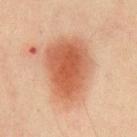Notes:
* follow-up: no biopsy performed (imaged during a skin exam)
* lesion diameter: ~7.5 mm (longest diameter)
* image: ~15 mm crop, total-body skin-cancer survey
* illumination: cross-polarized
* patient: male, aged around 60
* location: the chest